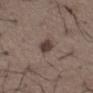<case>
<biopsy_status>not biopsied; imaged during a skin examination</biopsy_status>
<image>
  <source>total-body photography crop</source>
  <field_of_view_mm>15</field_of_view_mm>
</image>
<patient>
  <sex>male</sex>
  <age_approx>50</age_approx>
</patient>
<automated_metrics>
  <cielab_L>39</cielab_L>
  <cielab_a>13</cielab_a>
  <cielab_b>19</cielab_b>
</automated_metrics>
<lesion_size>
  <long_diameter_mm_approx>2.5</long_diameter_mm_approx>
</lesion_size>
<site>right thigh</site>
</case>